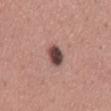Imaged during a routine full-body skin examination; the lesion was not biopsied and no histopathology is available.
The lesion is located on the mid back.
Automated tile analysis of the lesion measured a mean CIELAB color near L≈44 a*≈20 b*≈20, roughly 18 lightness units darker than nearby skin, and a normalized lesion–skin contrast near 13. The software also gave a border-irregularity rating of about 1.5/10, a color-variation rating of about 4/10, and peripheral color asymmetry of about 1. And it measured a nevus-likeness score of about 60/100 and a detector confidence of about 100 out of 100 that the crop contains a lesion.
The subject is a male aged 43–47.
This image is a 15 mm lesion crop taken from a total-body photograph.
Imaged with white-light lighting.
The recorded lesion diameter is about 3 mm.